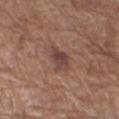Recorded during total-body skin imaging; not selected for excision or biopsy.
A region of skin cropped from a whole-body photographic capture, roughly 15 mm wide.
The subject is a male aged around 75.
Located on the arm.
Measured at roughly 3.5 mm in maximum diameter.
The total-body-photography lesion software estimated a lesion area of about 6 mm², a shape eccentricity near 0.75, and a symmetry-axis asymmetry near 0.2. And it measured a lesion color around L≈43 a*≈18 b*≈22 in CIELAB, about 9 CIELAB-L* units darker than the surrounding skin, and a normalized lesion–skin contrast near 7.5. The software also gave a border-irregularity index near 2/10, a within-lesion color-variation index near 4/10, and a peripheral color-asymmetry measure near 1.5.
The tile uses white-light illumination.